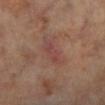Impression:
The lesion was tiled from a total-body skin photograph and was not biopsied.
Acquisition and patient details:
Captured under cross-polarized illumination. The lesion-visualizer software estimated an area of roughly 5 mm², an outline eccentricity of about 0.9 (0 = round, 1 = elongated), and two-axis asymmetry of about 0.2. The analysis additionally found an average lesion color of about L≈42 a*≈22 b*≈22 (CIELAB), roughly 6 lightness units darker than nearby skin, and a normalized border contrast of about 5. It also reported a color-variation rating of about 2/10 and peripheral color asymmetry of about 0.5. It also reported an automated nevus-likeness rating near 0 out of 100. The patient is a male roughly 70 years of age. A close-up tile cropped from a whole-body skin photograph, about 15 mm across. From the left lower leg. Approximately 4 mm at its widest.